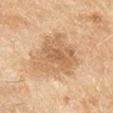Recorded during total-body skin imaging; not selected for excision or biopsy.
From the right lower leg.
A female subject, roughly 60 years of age.
Imaged with cross-polarized lighting.
A 15 mm crop from a total-body photograph taken for skin-cancer surveillance.